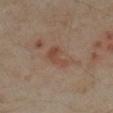A roughly 15 mm field-of-view crop from a total-body skin photograph.
Located on the leg.
The tile uses cross-polarized illumination.
About 3.5 mm across.
Automated image analysis of the tile measured a lesion area of about 5 mm² and two-axis asymmetry of about 0.45.
A female subject, in their mid- to late 30s.